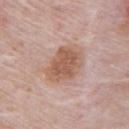This lesion was catalogued during total-body skin photography and was not selected for biopsy.
Cropped from a total-body skin-imaging series; the visible field is about 15 mm.
A male subject roughly 70 years of age.
Imaged with white-light lighting.
Longest diameter approximately 5 mm.
The lesion is located on the abdomen.